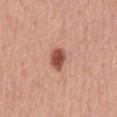notes: total-body-photography surveillance lesion; no biopsy
lesion diameter: ≈3.5 mm
image: total-body-photography crop, ~15 mm field of view
lighting: white-light
patient: male, aged approximately 70
site: the front of the torso